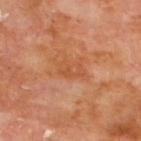biopsy status = imaged on a skin check; not biopsied.